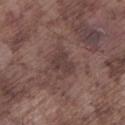Clinical impression: Recorded during total-body skin imaging; not selected for excision or biopsy. Acquisition and patient details: Located on the left lower leg. A male subject, aged 73 to 77. A 15 mm crop from a total-body photograph taken for skin-cancer surveillance. Approximately 3 mm at its widest. Captured under white-light illumination. Automated image analysis of the tile measured a within-lesion color-variation index near 1.5/10. The analysis additionally found an automated nevus-likeness rating near 0 out of 100 and lesion-presence confidence of about 100/100.